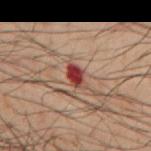<case>
  <biopsy_status>not biopsied; imaged during a skin examination</biopsy_status>
  <image>
    <source>total-body photography crop</source>
    <field_of_view_mm>15</field_of_view_mm>
  </image>
  <lesion_size>
    <long_diameter_mm_approx>2.5</long_diameter_mm_approx>
  </lesion_size>
  <automated_metrics>
    <border_irregularity_0_10>2.0</border_irregularity_0_10>
    <color_variation_0_10>3.5</color_variation_0_10>
  </automated_metrics>
  <patient>
    <sex>male</sex>
    <age_approx>40</age_approx>
  </patient>
  <site>left forearm</site>
</case>